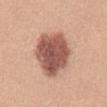workup: no biopsy performed (imaged during a skin exam) | tile lighting: white-light illumination | image source: ~15 mm tile from a whole-body skin photo | location: the front of the torso | patient: female, in their mid- to late 30s.